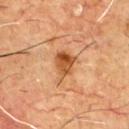This lesion was catalogued during total-body skin photography and was not selected for biopsy.
A 15 mm close-up tile from a total-body photography series done for melanoma screening.
The patient is a male aged 58–62.
The lesion's longest dimension is about 4 mm.
The lesion is located on the chest.
Captured under cross-polarized illumination.